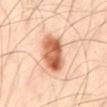{"biopsy_status": "not biopsied; imaged during a skin examination", "site": "mid back", "patient": {"sex": "male", "age_approx": 50}, "image": {"source": "total-body photography crop", "field_of_view_mm": 15}}